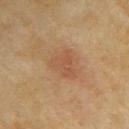notes — catalogued during a skin exam; not biopsied | subject — female, aged approximately 60 | anatomic site — the arm | image source — ~15 mm crop, total-body skin-cancer survey | diameter — about 3.5 mm.